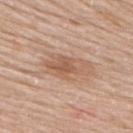| feature | finding |
|---|---|
| workup | total-body-photography surveillance lesion; no biopsy |
| subject | female, aged 53 to 57 |
| automated metrics | border irregularity of about 4.5 on a 0–10 scale, a color-variation rating of about 4.5/10, and peripheral color asymmetry of about 1.5 |
| imaging modality | 15 mm crop, total-body photography |
| anatomic site | the upper back |